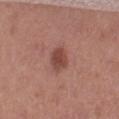{
  "biopsy_status": "not biopsied; imaged during a skin examination",
  "lesion_size": {
    "long_diameter_mm_approx": 3.5
  },
  "image": {
    "source": "total-body photography crop",
    "field_of_view_mm": 15
  },
  "automated_metrics": {
    "cielab_L": 46,
    "cielab_a": 24,
    "cielab_b": 25,
    "vs_skin_darker_L": 10.0,
    "border_irregularity_0_10": 2.0,
    "color_variation_0_10": 2.5,
    "peripheral_color_asymmetry": 1.0,
    "nevus_likeness_0_100": 95,
    "lesion_detection_confidence_0_100": 100
  },
  "patient": {
    "sex": "female",
    "age_approx": 40
  },
  "site": "right thigh"
}